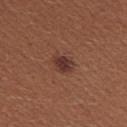{"site": "right upper arm", "lesion_size": {"long_diameter_mm_approx": 3.0}, "automated_metrics": {"area_mm2_approx": 5.5, "eccentricity": 0.65, "shape_asymmetry": 0.25, "cielab_L": 36, "cielab_a": 22, "cielab_b": 24, "vs_skin_darker_L": 9.0, "vs_skin_contrast_norm": 8.5, "color_variation_0_10": 3.0, "peripheral_color_asymmetry": 1.0, "lesion_detection_confidence_0_100": 100}, "lighting": "white-light", "patient": {"sex": "female", "age_approx": 25}, "image": {"source": "total-body photography crop", "field_of_view_mm": 15}}